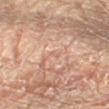Q: Is there a histopathology result?
A: no biopsy performed (imaged during a skin exam)
Q: What are the patient's age and sex?
A: male, in their mid- to late 50s
Q: Automated lesion metrics?
A: a footprint of about 1 mm², an outline eccentricity of about 0.9 (0 = round, 1 = elongated), and a shape-asymmetry score of about 0.4 (0 = symmetric); a mean CIELAB color near L≈64 a*≈22 b*≈29, about 5 CIELAB-L* units darker than the surrounding skin, and a lesion-to-skin contrast of about 3.5 (normalized; higher = more distinct); an automated nevus-likeness rating near 0 out of 100 and a detector confidence of about 0 out of 100 that the crop contains a lesion
Q: What lighting was used for the tile?
A: cross-polarized illumination
Q: How was this image acquired?
A: ~15 mm crop, total-body skin-cancer survey
Q: What is the anatomic site?
A: the arm
Q: What is the lesion's diameter?
A: ~1.5 mm (longest diameter)